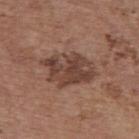follow-up = no biopsy performed (imaged during a skin exam)
tile lighting = white-light illumination
subject = female, roughly 65 years of age
lesion size = ≈6 mm
location = the upper back
image = ~15 mm crop, total-body skin-cancer survey